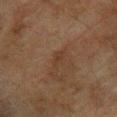<lesion>
  <biopsy_status>not biopsied; imaged during a skin examination</biopsy_status>
  <lesion_size>
    <long_diameter_mm_approx>3.0</long_diameter_mm_approx>
  </lesion_size>
  <image>
    <source>total-body photography crop</source>
    <field_of_view_mm>15</field_of_view_mm>
  </image>
  <automated_metrics>
    <cielab_L>30</cielab_L>
    <cielab_a>16</cielab_a>
    <cielab_b>25</cielab_b>
    <vs_skin_darker_L>5.0</vs_skin_darker_L>
    <vs_skin_contrast_norm>5.5</vs_skin_contrast_norm>
    <nevus_likeness_0_100>0</nevus_likeness_0_100>
    <lesion_detection_confidence_0_100>100</lesion_detection_confidence_0_100>
  </automated_metrics>
  <lighting>cross-polarized</lighting>
  <patient>
    <sex>male</sex>
    <age_approx>75</age_approx>
  </patient>
  <site>upper back</site>
</lesion>